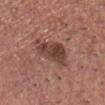Background: The recorded lesion diameter is about 5 mm. Automated image analysis of the tile measured a footprint of about 11 mm², an eccentricity of roughly 0.75, and two-axis asymmetry of about 0.3. The software also gave roughly 11 lightness units darker than nearby skin and a normalized border contrast of about 8.5. The analysis additionally found border irregularity of about 4 on a 0–10 scale and internal color variation of about 4.5 on a 0–10 scale. And it measured an automated nevus-likeness rating near 25 out of 100. A close-up tile cropped from a whole-body skin photograph, about 15 mm across. This is a white-light tile. Located on the head or neck. The patient is a male approximately 75 years of age.